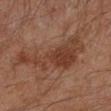Impression:
The lesion was photographed on a routine skin check and not biopsied; there is no pathology result.
Context:
Automated tile analysis of the lesion measured an area of roughly 23 mm² and an eccentricity of roughly 0.95. The software also gave a lesion color around L≈33 a*≈18 b*≈25 in CIELAB, about 7 CIELAB-L* units darker than the surrounding skin, and a normalized lesion–skin contrast near 7. It also reported border irregularity of about 8.5 on a 0–10 scale, a within-lesion color-variation index near 3.5/10, and a peripheral color-asymmetry measure near 1. The analysis additionally found a classifier nevus-likeness of about 20/100. The lesion's longest dimension is about 10 mm. Located on the arm. This image is a 15 mm lesion crop taken from a total-body photograph. The tile uses cross-polarized illumination. The patient is a male in their mid-40s.